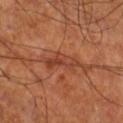Context: The lesion is located on the right lower leg. A 15 mm close-up tile from a total-body photography series done for melanoma screening. The subject is a male about 70 years old.